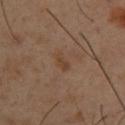Notes:
– follow-up · imaged on a skin check; not biopsied
– image source · ~15 mm tile from a whole-body skin photo
– anatomic site · the upper back
– patient · male, approximately 40 years of age